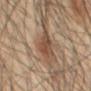biopsy status=imaged on a skin check; not biopsied
subject=male, in their 60s
acquisition=~15 mm tile from a whole-body skin photo
illumination=cross-polarized illumination
size=≈3 mm
automated metrics=an area of roughly 4.5 mm², a shape eccentricity near 0.7, and a symmetry-axis asymmetry near 0.15; a lesion color around L≈45 a*≈18 b*≈28 in CIELAB and a lesion–skin lightness drop of about 9; a border-irregularity rating of about 1.5/10, a within-lesion color-variation index near 3.5/10, and radial color variation of about 1.5; a nevus-likeness score of about 90/100 and a detector confidence of about 100 out of 100 that the crop contains a lesion
site=the abdomen